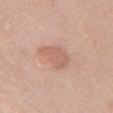Impression: Part of a total-body skin-imaging series; this lesion was reviewed on a skin check and was not flagged for biopsy. Context: Measured at roughly 4 mm in maximum diameter. Imaged with white-light lighting. A male subject, roughly 60 years of age. A 15 mm close-up extracted from a 3D total-body photography capture. Located on the chest.